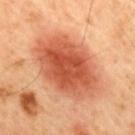<tbp_lesion>
  <biopsy_status>not biopsied; imaged during a skin examination</biopsy_status>
</tbp_lesion>